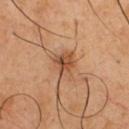notes = total-body-photography surveillance lesion; no biopsy | size = about 3.5 mm | patient = male, approximately 50 years of age | lighting = cross-polarized | imaging modality = total-body-photography crop, ~15 mm field of view | location = the front of the torso.